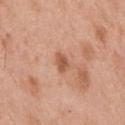{"biopsy_status": "not biopsied; imaged during a skin examination"}